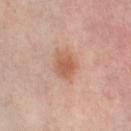Part of a total-body skin-imaging series; this lesion was reviewed on a skin check and was not flagged for biopsy.
The tile uses cross-polarized illumination.
The lesion is located on the right thigh.
A lesion tile, about 15 mm wide, cut from a 3D total-body photograph.
The lesion-visualizer software estimated a shape eccentricity near 0.7 and a shape-asymmetry score of about 0.15 (0 = symmetric). The software also gave a lesion color around L≈56 a*≈21 b*≈30 in CIELAB, roughly 9 lightness units darker than nearby skin, and a lesion-to-skin contrast of about 7 (normalized; higher = more distinct). The software also gave a border-irregularity rating of about 2/10, a within-lesion color-variation index near 4/10, and a peripheral color-asymmetry measure near 1. The analysis additionally found an automated nevus-likeness rating near 90 out of 100 and a lesion-detection confidence of about 100/100.
A female subject, aged approximately 50.
Longest diameter approximately 4 mm.